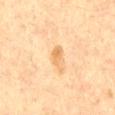Assessment: Part of a total-body skin-imaging series; this lesion was reviewed on a skin check and was not flagged for biopsy. Background: A roughly 15 mm field-of-view crop from a total-body skin photograph. The total-body-photography lesion software estimated an area of roughly 4.5 mm², an eccentricity of roughly 0.9, and a shape-asymmetry score of about 0.3 (0 = symmetric). The analysis additionally found a mean CIELAB color near L≈65 a*≈18 b*≈39, about 8 CIELAB-L* units darker than the surrounding skin, and a lesion-to-skin contrast of about 6 (normalized; higher = more distinct). And it measured radial color variation of about 1. Approximately 4 mm at its widest. A male subject aged 58 to 62.